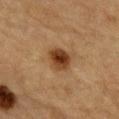biopsy_status: not biopsied; imaged during a skin examination
lighting: cross-polarized
lesion_size:
  long_diameter_mm_approx: 3.5
patient:
  sex: male
  age_approx: 85+
image:
  source: total-body photography crop
  field_of_view_mm: 15
site: abdomen